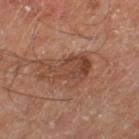Part of a total-body skin-imaging series; this lesion was reviewed on a skin check and was not flagged for biopsy. A lesion tile, about 15 mm wide, cut from a 3D total-body photograph. Imaged with cross-polarized lighting. An algorithmic analysis of the crop reported a footprint of about 12 mm² and an eccentricity of roughly 0.9. And it measured border irregularity of about 5.5 on a 0–10 scale, internal color variation of about 5 on a 0–10 scale, and peripheral color asymmetry of about 1.5. It also reported a classifier nevus-likeness of about 0/100. From the leg. A male subject, about 70 years old. Measured at roughly 5.5 mm in maximum diameter.